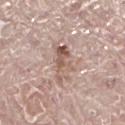Context:
On the right leg. A lesion tile, about 15 mm wide, cut from a 3D total-body photograph. A male subject aged around 70. Captured under white-light illumination.